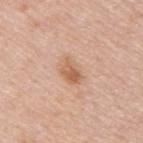Impression: The lesion was tiled from a total-body skin photograph and was not biopsied. Acquisition and patient details: Automated image analysis of the tile measured a lesion area of about 5.5 mm² and a shape-asymmetry score of about 0.2 (0 = symmetric). A region of skin cropped from a whole-body photographic capture, roughly 15 mm wide. The lesion is on the upper back. The subject is a male in their mid-40s. The lesion's longest dimension is about 3.5 mm.